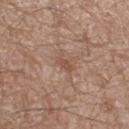Case summary:
• image source — total-body-photography crop, ~15 mm field of view
• body site — the left thigh
• subject — male, about 65 years old